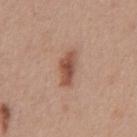Clinical impression:
Captured during whole-body skin photography for melanoma surveillance; the lesion was not biopsied.
Background:
The lesion is on the chest. A 15 mm close-up extracted from a 3D total-body photography capture. Approximately 4 mm at its widest. A male subject, aged around 30. Imaged with white-light lighting.